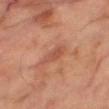Case summary:
* workup — imaged on a skin check; not biopsied
* acquisition — 15 mm crop, total-body photography
* patient — male, aged approximately 70
* body site — the right thigh
* illumination — cross-polarized illumination
* lesion size — about 3.5 mm